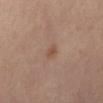Image and clinical context:
The subject is a female aged 48 to 52. About 2.5 mm across. Captured under cross-polarized illumination. Automated tile analysis of the lesion measured a mean CIELAB color near L≈48 a*≈18 b*≈28, about 6 CIELAB-L* units darker than the surrounding skin, and a normalized lesion–skin contrast near 6. The analysis additionally found a classifier nevus-likeness of about 35/100 and a detector confidence of about 100 out of 100 that the crop contains a lesion. From the mid back. A 15 mm crop from a total-body photograph taken for skin-cancer surveillance.